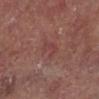  biopsy_status: not biopsied; imaged during a skin examination
  site: left lower leg
  lighting: white-light
  patient:
    sex: male
    age_approx: 80
  lesion_size:
    long_diameter_mm_approx: 3.0
  image:
    source: total-body photography crop
    field_of_view_mm: 15